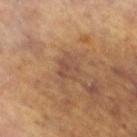{
  "biopsy_status": "not biopsied; imaged during a skin examination",
  "lighting": "cross-polarized",
  "patient": {
    "sex": "female",
    "age_approx": 65
  },
  "automated_metrics": {
    "area_mm2_approx": 5.0,
    "eccentricity": 0.4,
    "shape_asymmetry": 0.4,
    "cielab_L": 47,
    "cielab_a": 20,
    "cielab_b": 28,
    "vs_skin_darker_L": 6.0,
    "vs_skin_contrast_norm": 6.0,
    "lesion_detection_confidence_0_100": 90
  },
  "lesion_size": {
    "long_diameter_mm_approx": 2.5
  },
  "image": {
    "source": "total-body photography crop",
    "field_of_view_mm": 15
  },
  "site": "left leg"
}